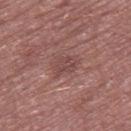Imaged during a routine full-body skin examination; the lesion was not biopsied and no histopathology is available.
A close-up tile cropped from a whole-body skin photograph, about 15 mm across.
The lesion is located on the right thigh.
The patient is a male aged approximately 55.